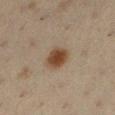No biopsy was performed on this lesion — it was imaged during a full skin examination and was not determined to be concerning. Automated tile analysis of the lesion measured a within-lesion color-variation index near 3/10 and peripheral color asymmetry of about 0.5. The analysis additionally found lesion-presence confidence of about 100/100. The lesion is on the left lower leg. A female subject approximately 40 years of age. A 15 mm close-up tile from a total-body photography series done for melanoma screening.